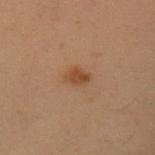{"biopsy_status": "not biopsied; imaged during a skin examination"}